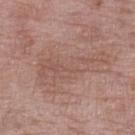The lesion was tiled from a total-body skin photograph and was not biopsied. The patient is a female about 70 years old. The lesion is located on the left lower leg. A close-up tile cropped from a whole-body skin photograph, about 15 mm across.